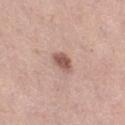The lesion was tiled from a total-body skin photograph and was not biopsied.
A female patient, about 60 years old.
About 3 mm across.
A roughly 15 mm field-of-view crop from a total-body skin photograph.
From the leg.
This is a white-light tile.
An algorithmic analysis of the crop reported a lesion area of about 4.5 mm², an eccentricity of roughly 0.7, and a symmetry-axis asymmetry near 0.25. The software also gave border irregularity of about 2 on a 0–10 scale and internal color variation of about 2.5 on a 0–10 scale. The software also gave lesion-presence confidence of about 100/100.